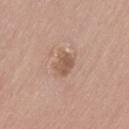Notes:
• biopsy status · total-body-photography surveillance lesion; no biopsy
• imaging modality · 15 mm crop, total-body photography
• subject · male, aged around 75
• anatomic site · the leg